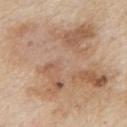The lesion's longest dimension is about 13.5 mm.
A male subject aged approximately 85 or older.
This image is a 15 mm lesion crop taken from a total-body photograph.
Imaged with white-light lighting.
Automated image analysis of the tile measured an area of roughly 105 mm² and a symmetry-axis asymmetry near 0.25. And it measured a mean CIELAB color near L≈62 a*≈16 b*≈31, roughly 9 lightness units darker than nearby skin, and a lesion-to-skin contrast of about 6 (normalized; higher = more distinct).
On the left upper arm.
Histopathologically confirmed as a melanoma in situ.